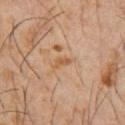<lesion>
<biopsy_status>not biopsied; imaged during a skin examination</biopsy_status>
<image>
  <source>total-body photography crop</source>
  <field_of_view_mm>15</field_of_view_mm>
</image>
<site>chest</site>
<patient>
  <sex>male</sex>
  <age_approx>60</age_approx>
</patient>
<automated_metrics>
  <area_mm2_approx>2.5</area_mm2_approx>
  <eccentricity>0.9</eccentricity>
  <shape_asymmetry>0.55</shape_asymmetry>
  <border_irregularity_0_10>5.0</border_irregularity_0_10>
  <color_variation_0_10>0.0</color_variation_0_10>
  <peripheral_color_asymmetry>0.0</peripheral_color_asymmetry>
  <nevus_likeness_0_100>0</nevus_likeness_0_100>
  <lesion_detection_confidence_0_100>100</lesion_detection_confidence_0_100>
</automated_metrics>
</lesion>